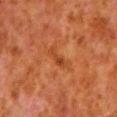biopsy_status: not biopsied; imaged during a skin examination
site: left lower leg
lighting: cross-polarized
patient:
  sex: male
  age_approx: 80
image:
  source: total-body photography crop
  field_of_view_mm: 15